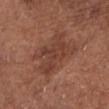workup = imaged on a skin check; not biopsied | image = ~15 mm tile from a whole-body skin photo | location = the chest | lesion diameter = ~6 mm (longest diameter) | subject = male, roughly 75 years of age | lighting = white-light illumination.